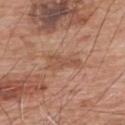Part of a total-body skin-imaging series; this lesion was reviewed on a skin check and was not flagged for biopsy.